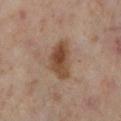The lesion was tiled from a total-body skin photograph and was not biopsied. The lesion is on the right lower leg. This image is a 15 mm lesion crop taken from a total-body photograph. A female patient, aged around 50.